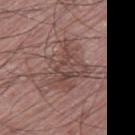biopsy status = total-body-photography surveillance lesion; no biopsy
illumination = white-light
patient = male, roughly 75 years of age
image = total-body-photography crop, ~15 mm field of view
diameter = ≈6 mm
location = the leg